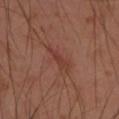<record>
  <biopsy_status>not biopsied; imaged during a skin examination</biopsy_status>
  <lesion_size>
    <long_diameter_mm_approx>4.0</long_diameter_mm_approx>
  </lesion_size>
  <patient>
    <sex>female</sex>
    <age_approx>55</age_approx>
  </patient>
  <lighting>cross-polarized</lighting>
  <automated_metrics>
    <border_irregularity_0_10>4.0</border_irregularity_0_10>
    <color_variation_0_10>0.5</color_variation_0_10>
    <peripheral_color_asymmetry>0.0</peripheral_color_asymmetry>
    <nevus_likeness_0_100>0</nevus_likeness_0_100>
    <lesion_detection_confidence_0_100>65</lesion_detection_confidence_0_100>
  </automated_metrics>
  <site>arm</site>
  <image>
    <source>total-body photography crop</source>
    <field_of_view_mm>15</field_of_view_mm>
  </image>
</record>